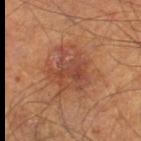biopsy_status: not biopsied; imaged during a skin examination
image:
  source: total-body photography crop
  field_of_view_mm: 15
site: left thigh
patient:
  sex: male
  age_approx: 70
lesion_size:
  long_diameter_mm_approx: 5.5
automated_metrics:
  cielab_L: 44
  cielab_a: 24
  cielab_b: 30
  vs_skin_darker_L: 8.0
  vs_skin_contrast_norm: 6.5
  border_irregularity_0_10: 4.5
  color_variation_0_10: 5.0
  peripheral_color_asymmetry: 1.5